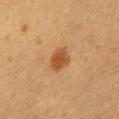Notes:
– notes — no biopsy performed (imaged during a skin exam)
– anatomic site — the mid back
– diameter — ~3.5 mm (longest diameter)
– patient — female, approximately 50 years of age
– image — total-body-photography crop, ~15 mm field of view
– lighting — cross-polarized illumination